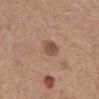notes: total-body-photography surveillance lesion; no biopsy | subject: male, aged around 75 | anatomic site: the chest | image source: ~15 mm crop, total-body skin-cancer survey | image-analysis metrics: a within-lesion color-variation index near 6.5/10 and a peripheral color-asymmetry measure near 2; an automated nevus-likeness rating near 65 out of 100 and a lesion-detection confidence of about 100/100 | size: about 4 mm | tile lighting: white-light.